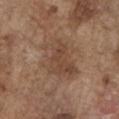Q: Is there a histopathology result?
A: total-body-photography surveillance lesion; no biopsy
Q: Automated lesion metrics?
A: an average lesion color of about L≈45 a*≈17 b*≈28 (CIELAB), a lesion–skin lightness drop of about 8, and a lesion-to-skin contrast of about 6 (normalized; higher = more distinct)
Q: What is the anatomic site?
A: the chest
Q: What kind of image is this?
A: total-body-photography crop, ~15 mm field of view
Q: What are the patient's age and sex?
A: male, aged approximately 75
Q: Lesion size?
A: ≈5.5 mm
Q: What lighting was used for the tile?
A: white-light illumination